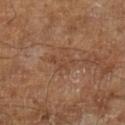Clinical impression: Part of a total-body skin-imaging series; this lesion was reviewed on a skin check and was not flagged for biopsy. Image and clinical context: A 15 mm close-up tile from a total-body photography series done for melanoma screening. A male subject, aged 63 to 67. The tile uses cross-polarized illumination. The lesion-visualizer software estimated a mean CIELAB color near L≈42 a*≈19 b*≈29, about 6 CIELAB-L* units darker than the surrounding skin, and a normalized border contrast of about 5. It also reported a border-irregularity index near 5.5/10, a color-variation rating of about 0/10, and radial color variation of about 0. It also reported a nevus-likeness score of about 0/100.